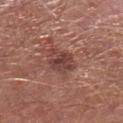Recorded during total-body skin imaging; not selected for excision or biopsy. A male subject, aged 63–67. Automated image analysis of the tile measured an outline eccentricity of about 0.45 (0 = round, 1 = elongated) and two-axis asymmetry of about 0.3. The analysis additionally found a lesion color around L≈42 a*≈23 b*≈23 in CIELAB, roughly 10 lightness units darker than nearby skin, and a normalized border contrast of about 8. The analysis additionally found an automated nevus-likeness rating near 5 out of 100 and lesion-presence confidence of about 100/100. The tile uses white-light illumination. On the left lower leg. Measured at roughly 3.5 mm in maximum diameter. Cropped from a total-body skin-imaging series; the visible field is about 15 mm.